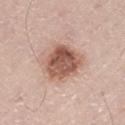Q: Was this lesion biopsied?
A: total-body-photography surveillance lesion; no biopsy